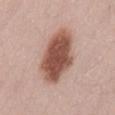Assessment: Part of a total-body skin-imaging series; this lesion was reviewed on a skin check and was not flagged for biopsy. Background: A male subject aged approximately 25. The lesion is located on the lower back. A close-up tile cropped from a whole-body skin photograph, about 15 mm across. Automated tile analysis of the lesion measured a footprint of about 23 mm², an eccentricity of roughly 0.75, and a shape-asymmetry score of about 0.15 (0 = symmetric). It also reported a lesion–skin lightness drop of about 17 and a lesion-to-skin contrast of about 11.5 (normalized; higher = more distinct). It also reported an automated nevus-likeness rating near 100 out of 100 and a lesion-detection confidence of about 100/100.